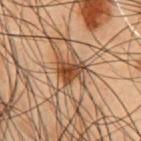workup = total-body-photography surveillance lesion; no biopsy
location = the front of the torso
lesion size = ~3.5 mm (longest diameter)
illumination = cross-polarized illumination
subject = male, about 55 years old
image = 15 mm crop, total-body photography